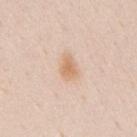lesion size: about 3 mm; subject: male, roughly 35 years of age; lighting: white-light; imaging modality: 15 mm crop, total-body photography; body site: the mid back.